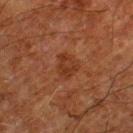Part of a total-body skin-imaging series; this lesion was reviewed on a skin check and was not flagged for biopsy. A 15 mm crop from a total-body photograph taken for skin-cancer surveillance. Imaged with cross-polarized lighting. Located on the left thigh. A male subject, aged 78–82. Automated image analysis of the tile measured a mean CIELAB color near L≈28 a*≈20 b*≈27 and roughly 5 lightness units darker than nearby skin. The software also gave a border-irregularity rating of about 2.5/10 and a peripheral color-asymmetry measure near 1.